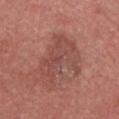follow-up: total-body-photography surveillance lesion; no biopsy | location: the head or neck | automated metrics: a border-irregularity index near 7/10, internal color variation of about 4 on a 0–10 scale, and radial color variation of about 1.5; a lesion-detection confidence of about 100/100 | imaging modality: 15 mm crop, total-body photography | size: ≈6 mm | patient: male, about 80 years old.